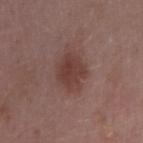  site: right upper arm
  patient:
    sex: female
    age_approx: 45
  image:
    source: total-body photography crop
    field_of_view_mm: 15
  lesion_size:
    long_diameter_mm_approx: 3.5
  lighting: white-light